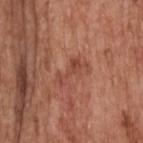The lesion was photographed on a routine skin check and not biopsied; there is no pathology result.
A region of skin cropped from a whole-body photographic capture, roughly 15 mm wide.
About 4 mm across.
The lesion is on the head or neck.
A male patient, aged 58 to 62.